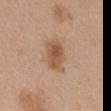{
  "biopsy_status": "not biopsied; imaged during a skin examination",
  "site": "mid back",
  "lesion_size": {
    "long_diameter_mm_approx": 5.0
  },
  "patient": {
    "sex": "female",
    "age_approx": 65
  },
  "automated_metrics": {
    "area_mm2_approx": 8.5,
    "eccentricity": 0.85,
    "shape_asymmetry": 0.3,
    "border_irregularity_0_10": 3.5,
    "color_variation_0_10": 4.0,
    "peripheral_color_asymmetry": 1.5,
    "nevus_likeness_0_100": 85
  },
  "image": {
    "source": "total-body photography crop",
    "field_of_view_mm": 15
  }
}